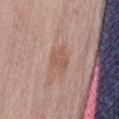Impression: Part of a total-body skin-imaging series; this lesion was reviewed on a skin check and was not flagged for biopsy. Image and clinical context: A female subject, aged 48 to 52. The lesion is located on the lower back. Automated image analysis of the tile measured an outline eccentricity of about 0.4 (0 = round, 1 = elongated) and two-axis asymmetry of about 0.3. And it measured a lesion color around L≈55 a*≈20 b*≈27 in CIELAB, roughly 7 lightness units darker than nearby skin, and a lesion-to-skin contrast of about 5.5 (normalized; higher = more distinct). The software also gave border irregularity of about 3 on a 0–10 scale, internal color variation of about 2 on a 0–10 scale, and a peripheral color-asymmetry measure near 0.5. The software also gave an automated nevus-likeness rating near 10 out of 100 and a lesion-detection confidence of about 100/100. Captured under white-light illumination. A lesion tile, about 15 mm wide, cut from a 3D total-body photograph. The recorded lesion diameter is about 2.5 mm.